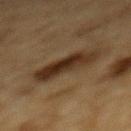workup: no biopsy performed (imaged during a skin exam)
lighting: cross-polarized illumination
image source: ~15 mm tile from a whole-body skin photo
anatomic site: the mid back
size: ≈5.5 mm
subject: male, in their mid- to late 80s or older